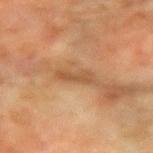biopsy_status: not biopsied; imaged during a skin examination
lesion_size:
  long_diameter_mm_approx: 3.5
image:
  source: total-body photography crop
  field_of_view_mm: 15
patient:
  sex: female
  age_approx: 55
lighting: cross-polarized
site: right forearm